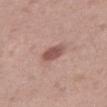The lesion was photographed on a routine skin check and not biopsied; there is no pathology result. A female patient, in their 40s. Approximately 3 mm at its widest. On the front of the torso. A close-up tile cropped from a whole-body skin photograph, about 15 mm across. The tile uses white-light illumination.